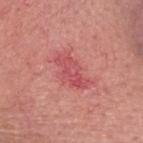Q: Is there a histopathology result?
A: imaged on a skin check; not biopsied
Q: Lesion size?
A: ~4.5 mm (longest diameter)
Q: What is the anatomic site?
A: the head or neck
Q: What kind of image is this?
A: total-body-photography crop, ~15 mm field of view
Q: How was the tile lit?
A: white-light illumination
Q: Automated lesion metrics?
A: a lesion area of about 7.5 mm²; a normalized border contrast of about 6
Q: Patient demographics?
A: male, aged approximately 80